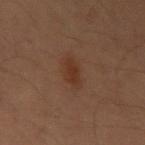The lesion was tiled from a total-body skin photograph and was not biopsied. Captured under cross-polarized illumination. About 3.5 mm across. A male patient, in their mid-30s. A region of skin cropped from a whole-body photographic capture, roughly 15 mm wide. The lesion is on the arm. An algorithmic analysis of the crop reported a footprint of about 6 mm² and an outline eccentricity of about 0.75 (0 = round, 1 = elongated). And it measured an average lesion color of about L≈30 a*≈18 b*≈26 (CIELAB) and a lesion-to-skin contrast of about 6.5 (normalized; higher = more distinct). It also reported a nevus-likeness score of about 70/100 and lesion-presence confidence of about 100/100.